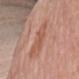Notes:
– notes: total-body-photography surveillance lesion; no biopsy
– image source: 15 mm crop, total-body photography
– automated lesion analysis: an area of roughly 7.5 mm² and a shape-asymmetry score of about 0.35 (0 = symmetric); border irregularity of about 5 on a 0–10 scale and radial color variation of about 0.5; a classifier nevus-likeness of about 0/100 and lesion-presence confidence of about 100/100
– subject: female, about 55 years old
– site: the right forearm